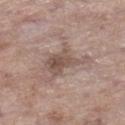patient — female, aged 83 or over
lesion diameter — ~5 mm (longest diameter)
TBP lesion metrics — a lesion area of about 10 mm² and an eccentricity of roughly 0.8
imaging modality — ~15 mm crop, total-body skin-cancer survey
body site — the right lower leg